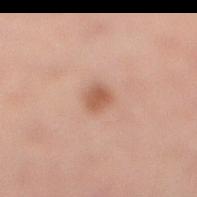The lesion was photographed on a routine skin check and not biopsied; there is no pathology result.
Cropped from a whole-body photographic skin survey; the tile spans about 15 mm.
The patient is a male aged 53 to 57.
From the leg.
The total-body-photography lesion software estimated a lesion color around L≈55 a*≈22 b*≈30 in CIELAB, about 10 CIELAB-L* units darker than the surrounding skin, and a normalized lesion–skin contrast near 7. The analysis additionally found a nevus-likeness score of about 85/100 and a detector confidence of about 100 out of 100 that the crop contains a lesion.
Measured at roughly 2.5 mm in maximum diameter.
The tile uses cross-polarized illumination.